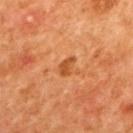Assessment: The lesion was photographed on a routine skin check and not biopsied; there is no pathology result. Image and clinical context: A female patient aged 38 to 42. A roughly 15 mm field-of-view crop from a total-body skin photograph. The lesion's longest dimension is about 2.5 mm. From the upper back. An algorithmic analysis of the crop reported a mean CIELAB color near L≈53 a*≈30 b*≈45, roughly 10 lightness units darker than nearby skin, and a lesion-to-skin contrast of about 7 (normalized; higher = more distinct). The analysis additionally found a border-irregularity index near 2.5/10, a color-variation rating of about 1/10, and radial color variation of about 0.5. The tile uses cross-polarized illumination.